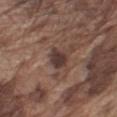The tile uses white-light illumination.
The lesion-visualizer software estimated a footprint of about 5 mm² and a shape-asymmetry score of about 0.25 (0 = symmetric). It also reported a classifier nevus-likeness of about 35/100.
A male subject about 75 years old.
A roughly 15 mm field-of-view crop from a total-body skin photograph.
From the arm.
Approximately 2.5 mm at its widest.